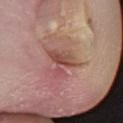Q: Was this lesion biopsied?
A: imaged on a skin check; not biopsied
Q: What kind of image is this?
A: ~15 mm tile from a whole-body skin photo
Q: Lesion location?
A: the right lower leg
Q: Illumination type?
A: white-light
Q: What is the lesion's diameter?
A: ~3.5 mm (longest diameter)
Q: Who is the patient?
A: female, in their mid-70s
Q: What did automated image analysis measure?
A: a mean CIELAB color near L≈49 a*≈25 b*≈25 and a lesion-to-skin contrast of about 7 (normalized; higher = more distinct); a border-irregularity index near 8/10 and a within-lesion color-variation index near 4.5/10